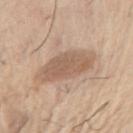Captured during whole-body skin photography for melanoma surveillance; the lesion was not biopsied. The lesion-visualizer software estimated a normalized lesion–skin contrast near 7. The analysis additionally found a border-irregularity rating of about 2.5/10, a within-lesion color-variation index near 3/10, and a peripheral color-asymmetry measure near 1. The analysis additionally found a classifier nevus-likeness of about 25/100 and a lesion-detection confidence of about 100/100. This is a white-light tile. A male patient, aged approximately 75. The recorded lesion diameter is about 7 mm. A lesion tile, about 15 mm wide, cut from a 3D total-body photograph. On the chest.